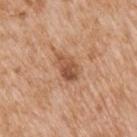follow-up=imaged on a skin check; not biopsied